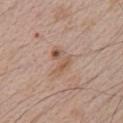Recorded during total-body skin imaging; not selected for excision or biopsy. This is a white-light tile. A male patient in their mid-60s. From the chest. Automated image analysis of the tile measured a lesion color around L≈56 a*≈18 b*≈29 in CIELAB and a normalized border contrast of about 6. Cropped from a total-body skin-imaging series; the visible field is about 15 mm. Longest diameter approximately 4 mm.